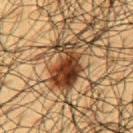The lesion was tiled from a total-body skin photograph and was not biopsied. The lesion is on the upper back. A roughly 15 mm field-of-view crop from a total-body skin photograph. A male patient in their 60s. The total-body-photography lesion software estimated a lesion color around L≈30 a*≈17 b*≈27 in CIELAB and a normalized border contrast of about 14. It also reported border irregularity of about 4 on a 0–10 scale, a color-variation rating of about 8.5/10, and peripheral color asymmetry of about 3. The analysis additionally found a classifier nevus-likeness of about 95/100 and lesion-presence confidence of about 100/100. About 5.5 mm across.